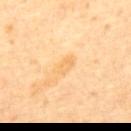Q: Was this lesion biopsied?
A: no biopsy performed (imaged during a skin exam)
Q: What is the anatomic site?
A: the mid back
Q: What is the imaging modality?
A: ~15 mm crop, total-body skin-cancer survey
Q: What lighting was used for the tile?
A: cross-polarized
Q: What are the patient's age and sex?
A: male, aged 58–62
Q: What is the lesion's diameter?
A: ≈3 mm
Q: Automated lesion metrics?
A: a footprint of about 3.5 mm², a shape eccentricity near 0.9, and a symmetry-axis asymmetry near 0.3; border irregularity of about 3 on a 0–10 scale, a color-variation rating of about 2.5/10, and a peripheral color-asymmetry measure near 1; an automated nevus-likeness rating near 0 out of 100 and lesion-presence confidence of about 100/100